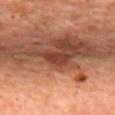Captured during whole-body skin photography for melanoma surveillance; the lesion was not biopsied. The lesion is on the upper back. The tile uses cross-polarized illumination. A 15 mm close-up extracted from a 3D total-body photography capture. A female subject aged 43 to 47.